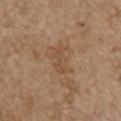Background:
The patient is a female aged around 65. From the front of the torso. A close-up tile cropped from a whole-body skin photograph, about 15 mm across. Measured at roughly 3.5 mm in maximum diameter.